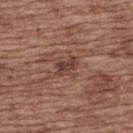Clinical impression:
The lesion was photographed on a routine skin check and not biopsied; there is no pathology result.
Context:
From the upper back. The subject is a female in their mid-70s. A lesion tile, about 15 mm wide, cut from a 3D total-body photograph.